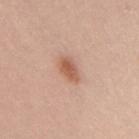notes — catalogued during a skin exam; not biopsied
body site — the upper back
automated lesion analysis — a classifier nevus-likeness of about 95/100 and lesion-presence confidence of about 100/100
patient — female, aged 28 to 32
image source — 15 mm crop, total-body photography
size — ≈3.5 mm